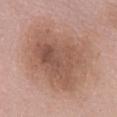This lesion was catalogued during total-body skin photography and was not selected for biopsy.
A 15 mm close-up extracted from a 3D total-body photography capture.
A female subject roughly 60 years of age.
On the mid back.